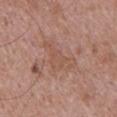Q: Was a biopsy performed?
A: no biopsy performed (imaged during a skin exam)
Q: What are the patient's age and sex?
A: male, aged 68 to 72
Q: How was this image acquired?
A: ~15 mm tile from a whole-body skin photo
Q: Lesion location?
A: the upper back